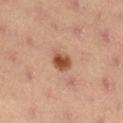<case>
  <biopsy_status>not biopsied; imaged during a skin examination</biopsy_status>
  <lesion_size>
    <long_diameter_mm_approx>2.5</long_diameter_mm_approx>
  </lesion_size>
  <lighting>cross-polarized</lighting>
  <patient>
    <sex>female</sex>
    <age_approx>35</age_approx>
  </patient>
  <site>right lower leg</site>
  <image>
    <source>total-body photography crop</source>
    <field_of_view_mm>15</field_of_view_mm>
  </image>
</case>